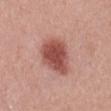Assessment: The lesion was tiled from a total-body skin photograph and was not biopsied. Context: The patient is a male about 55 years old. The lesion is on the mid back. Approximately 5 mm at its widest. Captured under white-light illumination. A region of skin cropped from a whole-body photographic capture, roughly 15 mm wide. The total-body-photography lesion software estimated a border-irregularity index near 2.5/10, a color-variation rating of about 3.5/10, and a peripheral color-asymmetry measure near 1.